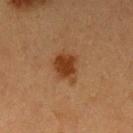Case summary:
• follow-up · total-body-photography surveillance lesion; no biopsy
• lesion size · ~3.5 mm (longest diameter)
• imaging modality · ~15 mm crop, total-body skin-cancer survey
• tile lighting · cross-polarized
• site · the arm
• subject · female, approximately 40 years of age